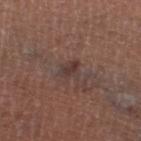Case summary:
• follow-up — total-body-photography surveillance lesion; no biopsy
• automated lesion analysis — a border-irregularity rating of about 3.5/10, a within-lesion color-variation index near 1.5/10, and peripheral color asymmetry of about 0.5; a classifier nevus-likeness of about 0/100 and lesion-presence confidence of about 90/100
• tile lighting — white-light
• patient — male, in their mid-60s
• image source — ~15 mm crop, total-body skin-cancer survey
• lesion diameter — ~3 mm (longest diameter)
• site — the right thigh